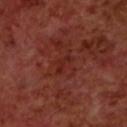<tbp_lesion>
<biopsy_status>not biopsied; imaged during a skin examination</biopsy_status>
<patient>
  <sex>male</sex>
  <age_approx>70</age_approx>
</patient>
<automated_metrics>
  <border_irregularity_0_10>5.5</border_irregularity_0_10>
  <nevus_likeness_0_100>0</nevus_likeness_0_100>
  <lesion_detection_confidence_0_100>100</lesion_detection_confidence_0_100>
</automated_metrics>
<lighting>cross-polarized</lighting>
<image>
  <source>total-body photography crop</source>
  <field_of_view_mm>15</field_of_view_mm>
</image>
<lesion_size>
  <long_diameter_mm_approx>2.5</long_diameter_mm_approx>
</lesion_size>
<site>upper back</site>
</tbp_lesion>